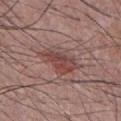The lesion was photographed on a routine skin check and not biopsied; there is no pathology result. The tile uses white-light illumination. A close-up tile cropped from a whole-body skin photograph, about 15 mm across. A male subject approximately 40 years of age. The recorded lesion diameter is about 5.5 mm. Automated tile analysis of the lesion measured a lesion area of about 11 mm² and a symmetry-axis asymmetry near 0.3. And it measured an automated nevus-likeness rating near 50 out of 100 and a detector confidence of about 100 out of 100 that the crop contains a lesion. The lesion is located on the chest.